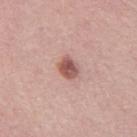Q: Was a biopsy performed?
A: total-body-photography surveillance lesion; no biopsy
Q: How was this image acquired?
A: ~15 mm tile from a whole-body skin photo
Q: What are the patient's age and sex?
A: female, aged 63–67
Q: Where on the body is the lesion?
A: the leg
Q: What did automated image analysis measure?
A: a footprint of about 5 mm², an outline eccentricity of about 0.6 (0 = round, 1 = elongated), and two-axis asymmetry of about 0.25; a mean CIELAB color near L≈55 a*≈23 b*≈24, roughly 14 lightness units darker than nearby skin, and a lesion-to-skin contrast of about 9 (normalized; higher = more distinct); a lesion-detection confidence of about 100/100
Q: How was the tile lit?
A: white-light illumination